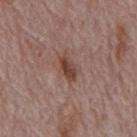This lesion was catalogued during total-body skin photography and was not selected for biopsy.
On the back.
Imaged with white-light lighting.
Cropped from a total-body skin-imaging series; the visible field is about 15 mm.
A male patient, aged 68–72.